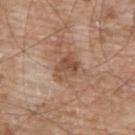Imaged during a routine full-body skin examination; the lesion was not biopsied and no histopathology is available. A male patient, aged approximately 65. Located on the upper back. Measured at roughly 3 mm in maximum diameter. A 15 mm crop from a total-body photograph taken for skin-cancer surveillance.